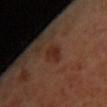biopsy status: catalogued during a skin exam; not biopsied | location: the chest | size: about 2.5 mm | illumination: cross-polarized | image source: 15 mm crop, total-body photography | image-analysis metrics: a nevus-likeness score of about 65/100 and lesion-presence confidence of about 100/100 | subject: female, aged 38–42.